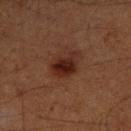Recorded during total-body skin imaging; not selected for excision or biopsy.
The patient is a male aged 58 to 62.
A 15 mm close-up tile from a total-body photography series done for melanoma screening.
The recorded lesion diameter is about 4 mm.
The lesion is located on the left lower leg.
Captured under cross-polarized illumination.